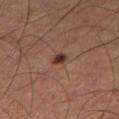Q: Is there a histopathology result?
A: total-body-photography surveillance lesion; no biopsy
Q: Where on the body is the lesion?
A: the right thigh
Q: Automated lesion metrics?
A: an area of roughly 2.5 mm² and an outline eccentricity of about 0.75 (0 = round, 1 = elongated); an average lesion color of about L≈31 a*≈19 b*≈22 (CIELAB), about 12 CIELAB-L* units darker than the surrounding skin, and a normalized lesion–skin contrast near 11; border irregularity of about 2.5 on a 0–10 scale and a peripheral color-asymmetry measure near 0.5; a nevus-likeness score of about 95/100 and lesion-presence confidence of about 100/100
Q: What are the patient's age and sex?
A: male, roughly 60 years of age
Q: How was the tile lit?
A: cross-polarized
Q: What is the imaging modality?
A: 15 mm crop, total-body photography